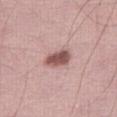| key | value |
|---|---|
| notes | no biopsy performed (imaged during a skin exam) |
| subject | male, aged 43–47 |
| automated metrics | an area of roughly 6.5 mm² and two-axis asymmetry of about 0.2; a border-irregularity rating of about 2/10, a within-lesion color-variation index near 3/10, and peripheral color asymmetry of about 1; an automated nevus-likeness rating near 90 out of 100 |
| location | the right thigh |
| imaging modality | 15 mm crop, total-body photography |
| lighting | white-light illumination |
| diameter | ~3.5 mm (longest diameter) |